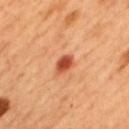Located on the mid back.
A male patient approximately 50 years of age.
Automated tile analysis of the lesion measured a classifier nevus-likeness of about 95/100 and a lesion-detection confidence of about 100/100.
The tile uses cross-polarized illumination.
A lesion tile, about 15 mm wide, cut from a 3D total-body photograph.
Approximately 3 mm at its widest.